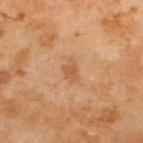This lesion was catalogued during total-body skin photography and was not selected for biopsy.
Approximately 2.5 mm at its widest.
The tile uses cross-polarized illumination.
From the upper back.
A roughly 15 mm field-of-view crop from a total-body skin photograph.
The patient is a male about 70 years old.